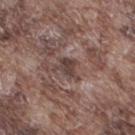Captured during whole-body skin photography for melanoma surveillance; the lesion was not biopsied. A lesion tile, about 15 mm wide, cut from a 3D total-body photograph. Located on the right thigh. The tile uses white-light illumination. The patient is a male aged 73–77.